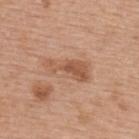workup — total-body-photography surveillance lesion; no biopsy
acquisition — ~15 mm crop, total-body skin-cancer survey
subject — female, in their mid- to late 60s
size — about 5.5 mm
body site — the upper back